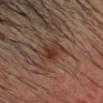Imaged during a routine full-body skin examination; the lesion was not biopsied and no histopathology is available. A 15 mm crop from a total-body photograph taken for skin-cancer surveillance. On the head or neck. A male patient, about 35 years old.